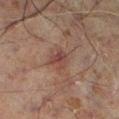patient — male, about 60 years old | imaging modality — 15 mm crop, total-body photography | location — the left lower leg.